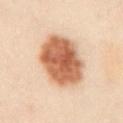Q: Was a biopsy performed?
A: imaged on a skin check; not biopsied
Q: Where on the body is the lesion?
A: the abdomen
Q: Illumination type?
A: cross-polarized illumination
Q: What are the patient's age and sex?
A: male, aged 48–52
Q: How large is the lesion?
A: about 6.5 mm
Q: Automated lesion metrics?
A: a shape eccentricity near 0.55 and a shape-asymmetry score of about 0.1 (0 = symmetric); a detector confidence of about 100 out of 100 that the crop contains a lesion
Q: What kind of image is this?
A: ~15 mm crop, total-body skin-cancer survey